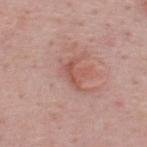Clinical summary:
Measured at roughly 3.5 mm in maximum diameter. A 15 mm crop from a total-body photograph taken for skin-cancer surveillance. Captured under white-light illumination. A male subject aged approximately 50. Located on the back.